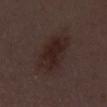Case summary:
• follow-up: imaged on a skin check; not biopsied
• subject: male, aged 53 to 57
• illumination: white-light
• body site: the front of the torso
• diameter: about 6.5 mm
• image: ~15 mm tile from a whole-body skin photo